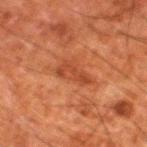Impression:
Recorded during total-body skin imaging; not selected for excision or biopsy.
Context:
This is a cross-polarized tile. Measured at roughly 4 mm in maximum diameter. The lesion-visualizer software estimated a mean CIELAB color near L≈36 a*≈25 b*≈32 and a lesion–skin lightness drop of about 7. It also reported a border-irregularity index near 4.5/10 and a peripheral color-asymmetry measure near 0.5. The analysis additionally found an automated nevus-likeness rating near 0 out of 100 and lesion-presence confidence of about 100/100. From the left lower leg. A male patient, aged 78 to 82. Cropped from a total-body skin-imaging series; the visible field is about 15 mm.